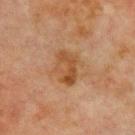Part of a total-body skin-imaging series; this lesion was reviewed on a skin check and was not flagged for biopsy. A close-up tile cropped from a whole-body skin photograph, about 15 mm across. The patient is a male about 70 years old. Located on the chest. Imaged with cross-polarized lighting.